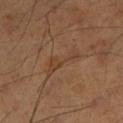Acquisition and patient details:
The tile uses cross-polarized illumination. Longest diameter approximately 4.5 mm. Located on the left lower leg. The subject is a male aged 53 to 57. Cropped from a total-body skin-imaging series; the visible field is about 15 mm.